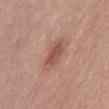The lesion is on the abdomen. The lesion's longest dimension is about 4 mm. A male patient approximately 65 years of age. A region of skin cropped from a whole-body photographic capture, roughly 15 mm wide.